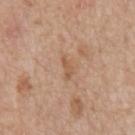The lesion was tiled from a total-body skin photograph and was not biopsied.
A male patient, aged approximately 65.
Captured under white-light illumination.
The recorded lesion diameter is about 2.5 mm.
A 15 mm crop from a total-body photograph taken for skin-cancer surveillance.
The lesion is located on the chest.